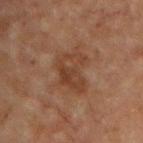workup=total-body-photography surveillance lesion; no biopsy